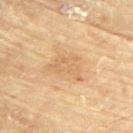The lesion was tiled from a total-body skin photograph and was not biopsied.
The subject is a female aged around 80.
Automated image analysis of the tile measured an outline eccentricity of about 0.8 (0 = round, 1 = elongated) and a symmetry-axis asymmetry near 0.55. The software also gave lesion-presence confidence of about 100/100.
A lesion tile, about 15 mm wide, cut from a 3D total-body photograph.
The lesion is located on the upper back.
The recorded lesion diameter is about 5 mm.
Captured under cross-polarized illumination.